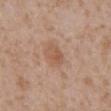follow-up = catalogued during a skin exam; not biopsied | automated lesion analysis = a lesion color around L≈55 a*≈20 b*≈30 in CIELAB, about 8 CIELAB-L* units darker than the surrounding skin, and a normalized lesion–skin contrast near 5.5; a color-variation rating of about 3/10 and a peripheral color-asymmetry measure near 1; a classifier nevus-likeness of about 5/100 | anatomic site = the abdomen | subject = male, aged 63–67 | lesion size = ~3 mm (longest diameter) | image source = ~15 mm tile from a whole-body skin photo.